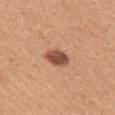  biopsy_status: not biopsied; imaged during a skin examination
  lesion_size:
    long_diameter_mm_approx: 3.5
  image:
    source: total-body photography crop
    field_of_view_mm: 15
  site: back
  patient:
    sex: female
    age_approx: 30